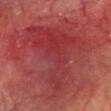Findings:
– biopsy status · catalogued during a skin exam; not biopsied
– size · ~14.5 mm (longest diameter)
– lighting · cross-polarized
– body site · the head or neck
– patient · male, aged around 75
– TBP lesion metrics · a lesion area of about 100 mm², a shape eccentricity near 0.4, and two-axis asymmetry of about 0.4; a mean CIELAB color near L≈34 a*≈31 b*≈22 and a lesion-to-skin contrast of about 6.5 (normalized; higher = more distinct)
– imaging modality · ~15 mm crop, total-body skin-cancer survey